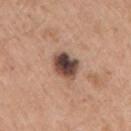<lesion>
<biopsy_status>not biopsied; imaged during a skin examination</biopsy_status>
<patient>
  <sex>male</sex>
  <age_approx>60</age_approx>
</patient>
<site>right upper arm</site>
<image>
  <source>total-body photography crop</source>
  <field_of_view_mm>15</field_of_view_mm>
</image>
<lesion_size>
  <long_diameter_mm_approx>3.5</long_diameter_mm_approx>
</lesion_size>
<lighting>white-light</lighting>
</lesion>